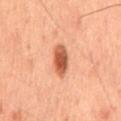Notes:
- follow-up: catalogued during a skin exam; not biopsied
- subject: male, aged approximately 60
- automated metrics: border irregularity of about 1 on a 0–10 scale, a color-variation rating of about 4.5/10, and peripheral color asymmetry of about 1.5
- anatomic site: the mid back
- image source: total-body-photography crop, ~15 mm field of view